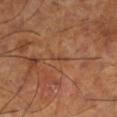Notes:
* notes · no biopsy performed (imaged during a skin exam)
* tile lighting · cross-polarized illumination
* site · the left lower leg
* image · 15 mm crop, total-body photography
* automated metrics · roughly 6 lightness units darker than nearby skin and a lesion-to-skin contrast of about 5.5 (normalized; higher = more distinct)
* subject · male, in their mid-60s